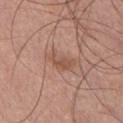The lesion was photographed on a routine skin check and not biopsied; there is no pathology result. Longest diameter approximately 4 mm. A region of skin cropped from a whole-body photographic capture, roughly 15 mm wide. Automated tile analysis of the lesion measured an average lesion color of about L≈53 a*≈21 b*≈28 (CIELAB), about 8 CIELAB-L* units darker than the surrounding skin, and a lesion-to-skin contrast of about 6 (normalized; higher = more distinct). The analysis additionally found a nevus-likeness score of about 0/100. Captured under white-light illumination. Located on the chest. The subject is a male roughly 30 years of age.